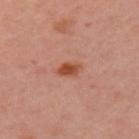  biopsy_status: not biopsied; imaged during a skin examination
  automated_metrics:
    eccentricity: 0.8
    shape_asymmetry: 0.2
    nevus_likeness_0_100: 100
    lesion_detection_confidence_0_100: 100
  image:
    source: total-body photography crop
    field_of_view_mm: 15
  site: left upper arm
  lighting: cross-polarized
  patient:
    sex: female
    age_approx: 40